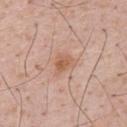size: ~3 mm (longest diameter)
image source: total-body-photography crop, ~15 mm field of view
automated lesion analysis: a classifier nevus-likeness of about 35/100
subject: male, aged approximately 55
site: the back
lighting: white-light illumination